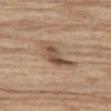notes: imaged on a skin check; not biopsied
body site: the left thigh
tile lighting: white-light illumination
size: ~5 mm (longest diameter)
patient: male, aged approximately 70
image: total-body-photography crop, ~15 mm field of view